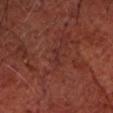| feature | finding |
|---|---|
| workup | total-body-photography surveillance lesion; no biopsy |
| location | the head or neck |
| tile lighting | cross-polarized illumination |
| image | ~15 mm crop, total-body skin-cancer survey |
| patient | about 65 years old |